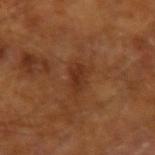The lesion was tiled from a total-body skin photograph and was not biopsied.
From the left forearm.
Approximately 3 mm at its widest.
Captured under cross-polarized illumination.
A male patient aged 63 to 67.
This image is a 15 mm lesion crop taken from a total-body photograph.